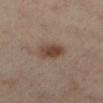Case summary:
• notes: total-body-photography surveillance lesion; no biopsy
• lesion diameter: ≈3.5 mm
• anatomic site: the left lower leg
• subject: female, aged around 50
• imaging modality: ~15 mm crop, total-body skin-cancer survey